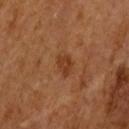Part of a total-body skin-imaging series; this lesion was reviewed on a skin check and was not flagged for biopsy. The patient is a male in their mid- to late 60s. A close-up tile cropped from a whole-body skin photograph, about 15 mm across. Located on the left upper arm.